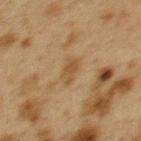Notes:
– notes — catalogued during a skin exam; not biopsied